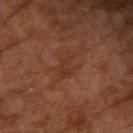The lesion was photographed on a routine skin check and not biopsied; there is no pathology result. A 15 mm close-up extracted from a 3D total-body photography capture. The lesion is located on the right upper arm. A male subject aged approximately 55.